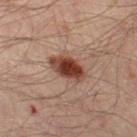  biopsy_status: not biopsied; imaged during a skin examination
  image:
    source: total-body photography crop
    field_of_view_mm: 15
  patient:
    sex: male
    age_approx: 45
  site: left thigh